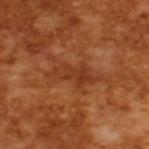- notes: no biopsy performed (imaged during a skin exam)
- subject: male, aged approximately 65
- image source: total-body-photography crop, ~15 mm field of view